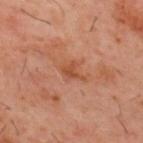Recorded during total-body skin imaging; not selected for excision or biopsy. The subject is a male aged around 60. A region of skin cropped from a whole-body photographic capture, roughly 15 mm wide. From the mid back.